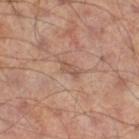biopsy_status: not biopsied; imaged during a skin examination
lesion_size:
  long_diameter_mm_approx: 2.5
lighting: cross-polarized
automated_metrics:
  eccentricity: 0.9
  shape_asymmetry: 0.4
  cielab_L: 50
  cielab_a: 19
  cielab_b: 27
  vs_skin_darker_L: 7.0
  border_irregularity_0_10: 4.0
  peripheral_color_asymmetry: 0.0
  nevus_likeness_0_100: 0
  lesion_detection_confidence_0_100: 95
image:
  source: total-body photography crop
  field_of_view_mm: 15
patient:
  sex: male
  age_approx: 65
site: leg